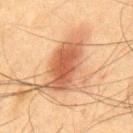biopsy status = total-body-photography surveillance lesion; no biopsy
image = 15 mm crop, total-body photography
subject = male, roughly 45 years of age
body site = the mid back
lesion size = about 9 mm